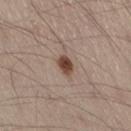Recorded during total-body skin imaging; not selected for excision or biopsy.
Captured under white-light illumination.
The lesion's longest dimension is about 3 mm.
The lesion is located on the right thigh.
Automated tile analysis of the lesion measured a footprint of about 4 mm², an eccentricity of roughly 0.75, and a symmetry-axis asymmetry near 0.15. The software also gave a mean CIELAB color near L≈45 a*≈17 b*≈26, roughly 14 lightness units darker than nearby skin, and a normalized lesion–skin contrast near 10.5. The software also gave a border-irregularity index near 1.5/10, internal color variation of about 3 on a 0–10 scale, and radial color variation of about 1.
This image is a 15 mm lesion crop taken from a total-body photograph.
The subject is a male roughly 55 years of age.